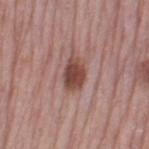Case summary:
- follow-up — imaged on a skin check; not biopsied
- location — the left thigh
- lesion diameter — ~3.5 mm (longest diameter)
- TBP lesion metrics — an eccentricity of roughly 0.75 and a symmetry-axis asymmetry near 0.15; a border-irregularity index near 1.5/10 and peripheral color asymmetry of about 1; a classifier nevus-likeness of about 95/100 and a detector confidence of about 100 out of 100 that the crop contains a lesion
- imaging modality — total-body-photography crop, ~15 mm field of view
- subject — female, aged 63–67